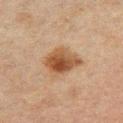{"biopsy_status": "not biopsied; imaged during a skin examination", "lighting": "cross-polarized", "automated_metrics": {"eccentricity": 0.4, "shape_asymmetry": 0.3, "nevus_likeness_0_100": 95, "lesion_detection_confidence_0_100": 100}, "site": "left upper arm", "image": {"source": "total-body photography crop", "field_of_view_mm": 15}, "patient": {"sex": "male", "age_approx": 50}}